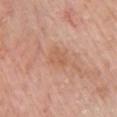{
  "biopsy_status": "not biopsied; imaged during a skin examination",
  "patient": {
    "sex": "male",
    "age_approx": 80
  },
  "image": {
    "source": "total-body photography crop",
    "field_of_view_mm": 15
  },
  "site": "front of the torso"
}